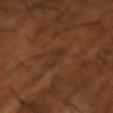<record>
<lesion_size>
  <long_diameter_mm_approx>2.5</long_diameter_mm_approx>
</lesion_size>
<automated_metrics>
  <area_mm2_approx>3.5</area_mm2_approx>
  <eccentricity>0.75</eccentricity>
  <shape_asymmetry>0.55</shape_asymmetry>
  <cielab_L>30</cielab_L>
  <cielab_a>20</cielab_a>
  <cielab_b>29</cielab_b>
  <border_irregularity_0_10>5.5</border_irregularity_0_10>
  <color_variation_0_10>1.0</color_variation_0_10>
  <peripheral_color_asymmetry>0.5</peripheral_color_asymmetry>
</automated_metrics>
<image>
  <source>total-body photography crop</source>
  <field_of_view_mm>15</field_of_view_mm>
</image>
<patient>
  <sex>male</sex>
  <age_approx>65</age_approx>
</patient>
<site>right forearm</site>
<lighting>cross-polarized</lighting>
</record>